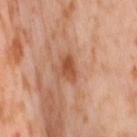Notes:
* workup — imaged on a skin check; not biopsied
* subject — female, in their mid-50s
* imaging modality — ~15 mm crop, total-body skin-cancer survey
* diameter — about 3 mm
* site — the right thigh